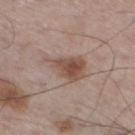This lesion was catalogued during total-body skin photography and was not selected for biopsy.
Located on the leg.
Captured under white-light illumination.
A 15 mm close-up tile from a total-body photography series done for melanoma screening.
A male subject, aged 58 to 62.